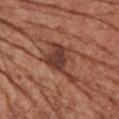Part of a total-body skin-imaging series; this lesion was reviewed on a skin check and was not flagged for biopsy.
Located on the front of the torso.
The subject is a female aged 73–77.
A 15 mm close-up tile from a total-body photography series done for melanoma screening.
Automated tile analysis of the lesion measured a nevus-likeness score of about 15/100 and a detector confidence of about 100 out of 100 that the crop contains a lesion.
Measured at roughly 6 mm in maximum diameter.